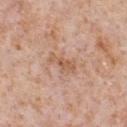The lesion was photographed on a routine skin check and not biopsied; there is no pathology result. Imaged with white-light lighting. A male subject, aged 63 to 67. A lesion tile, about 15 mm wide, cut from a 3D total-body photograph. The total-body-photography lesion software estimated a lesion area of about 4 mm², an eccentricity of roughly 0.9, and a symmetry-axis asymmetry near 0.35. It also reported a border-irregularity index near 4/10, internal color variation of about 1 on a 0–10 scale, and radial color variation of about 0.5. The recorded lesion diameter is about 3.5 mm. Located on the front of the torso.